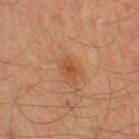Assessment: Recorded during total-body skin imaging; not selected for excision or biopsy. Context: Approximately 3 mm at its widest. The lesion is on the right thigh. A 15 mm close-up tile from a total-body photography series done for melanoma screening. This is a cross-polarized tile. The total-body-photography lesion software estimated border irregularity of about 3 on a 0–10 scale, a within-lesion color-variation index near 2.5/10, and radial color variation of about 1. A male subject, aged 38 to 42.